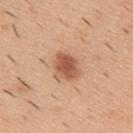Clinical impression:
No biopsy was performed on this lesion — it was imaged during a full skin examination and was not determined to be concerning.
Image and clinical context:
Cropped from a whole-body photographic skin survey; the tile spans about 15 mm. From the upper back. The patient is a male aged approximately 40.